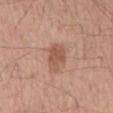Recorded during total-body skin imaging; not selected for excision or biopsy. The lesion is located on the lower back. The lesion's longest dimension is about 3.5 mm. A close-up tile cropped from a whole-body skin photograph, about 15 mm across. Automated tile analysis of the lesion measured a lesion area of about 7 mm². Captured under white-light illumination. The subject is a male aged 53–57.